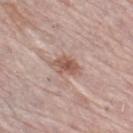This lesion was catalogued during total-body skin photography and was not selected for biopsy.
Automated image analysis of the tile measured an area of roughly 6.5 mm², an outline eccentricity of about 0.8 (0 = round, 1 = elongated), and two-axis asymmetry of about 0.3. It also reported roughly 10 lightness units darker than nearby skin and a normalized lesion–skin contrast near 7.5. And it measured a border-irregularity rating of about 3/10, a color-variation rating of about 4.5/10, and a peripheral color-asymmetry measure near 1.5.
This is a white-light tile.
The lesion is located on the left leg.
A female subject, aged around 65.
A region of skin cropped from a whole-body photographic capture, roughly 15 mm wide.